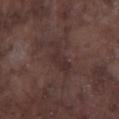notes: no biopsy performed (imaged during a skin exam)
location: the left lower leg
image: total-body-photography crop, ~15 mm field of view
lesion size: about 4.5 mm
TBP lesion metrics: a lesion color around L≈30 a*≈16 b*≈16 in CIELAB and a lesion–skin lightness drop of about 5; a border-irregularity rating of about 5.5/10, a color-variation rating of about 1.5/10, and peripheral color asymmetry of about 0.5
patient: male, aged 73–77
illumination: white-light